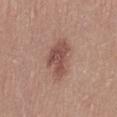biopsy status = no biopsy performed (imaged during a skin exam).